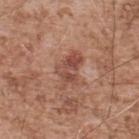Q: What did automated image analysis measure?
A: an average lesion color of about L≈49 a*≈23 b*≈28 (CIELAB) and a lesion-to-skin contrast of about 7 (normalized; higher = more distinct)
Q: What are the patient's age and sex?
A: male, aged 53–57
Q: Illumination type?
A: white-light illumination
Q: How was this image acquired?
A: ~15 mm crop, total-body skin-cancer survey
Q: Where on the body is the lesion?
A: the upper back